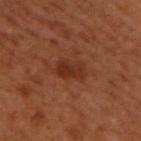Assessment: The lesion was photographed on a routine skin check and not biopsied; there is no pathology result. Background: The patient is a male aged around 50. The tile uses cross-polarized illumination. A 15 mm close-up tile from a total-body photography series done for melanoma screening. Located on the upper back. Approximately 3.5 mm at its widest.